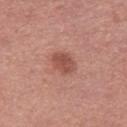This lesion was catalogued during total-body skin photography and was not selected for biopsy. An algorithmic analysis of the crop reported a lesion area of about 5.5 mm², an outline eccentricity of about 0.7 (0 = round, 1 = elongated), and two-axis asymmetry of about 0.2. The analysis additionally found a border-irregularity index near 2/10, a color-variation rating of about 2.5/10, and peripheral color asymmetry of about 1. The analysis additionally found a nevus-likeness score of about 70/100 and lesion-presence confidence of about 100/100. The tile uses white-light illumination. A female subject aged approximately 30. The lesion is located on the left thigh. A 15 mm close-up tile from a total-body photography series done for melanoma screening. The recorded lesion diameter is about 3 mm.